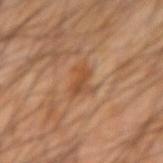* biopsy status — imaged on a skin check; not biopsied
* size — ≈3 mm
* patient — male, approximately 45 years of age
* anatomic site — the left forearm
* illumination — cross-polarized
* image source — ~15 mm crop, total-body skin-cancer survey
* automated metrics — a footprint of about 5 mm², an eccentricity of roughly 0.7, and a symmetry-axis asymmetry near 0.45; a normalized lesion–skin contrast near 6; a lesion-detection confidence of about 100/100